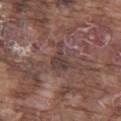notes = no biopsy performed (imaged during a skin exam) | anatomic site = the left thigh | imaging modality = total-body-photography crop, ~15 mm field of view | lesion size = ≈3.5 mm | subject = male, in their mid- to late 70s | lighting = white-light illumination | image-analysis metrics = a border-irregularity index near 6.5/10, a within-lesion color-variation index near 2/10, and peripheral color asymmetry of about 1; a lesion-detection confidence of about 50/100.